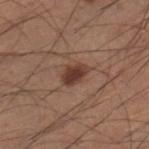  site: leg
  lighting: white-light
  automated_metrics:
    border_irregularity_0_10: 2.0
    color_variation_0_10: 3.0
    peripheral_color_asymmetry: 1.0
  image:
    source: total-body photography crop
    field_of_view_mm: 15
  patient:
    sex: male
    age_approx: 35